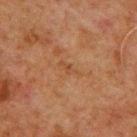biopsy status: total-body-photography surveillance lesion; no biopsy | anatomic site: the upper back | illumination: cross-polarized | patient: male, approximately 60 years of age | imaging modality: ~15 mm tile from a whole-body skin photo | size: ~2.5 mm (longest diameter).